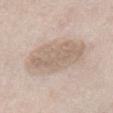Clinical impression:
Captured during whole-body skin photography for melanoma surveillance; the lesion was not biopsied.
Context:
A male subject in their mid-70s. The lesion-visualizer software estimated a footprint of about 24 mm², an outline eccentricity of about 0.65 (0 = round, 1 = elongated), and a shape-asymmetry score of about 0.15 (0 = symmetric). The software also gave roughly 9 lightness units darker than nearby skin and a lesion-to-skin contrast of about 6 (normalized; higher = more distinct). A 15 mm close-up tile from a total-body photography series done for melanoma screening. On the front of the torso. Longest diameter approximately 6 mm.